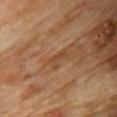Recorded during total-body skin imaging; not selected for excision or biopsy. The lesion's longest dimension is about 2.5 mm. Captured under cross-polarized illumination. A roughly 15 mm field-of-view crop from a total-body skin photograph. The subject is a female aged approximately 80. On the chest.